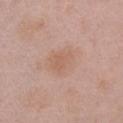Clinical impression: Part of a total-body skin-imaging series; this lesion was reviewed on a skin check and was not flagged for biopsy. Acquisition and patient details: From the arm. A 15 mm close-up tile from a total-body photography series done for melanoma screening. A male patient, aged around 50.